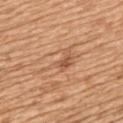{"biopsy_status": "not biopsied; imaged during a skin examination", "site": "back", "image": {"source": "total-body photography crop", "field_of_view_mm": 15}, "patient": {"sex": "female", "age_approx": 55}, "lighting": "white-light"}